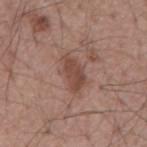Impression:
No biopsy was performed on this lesion — it was imaged during a full skin examination and was not determined to be concerning.
Image and clinical context:
On the mid back. A male subject, aged 53 to 57. Cropped from a whole-body photographic skin survey; the tile spans about 15 mm. An algorithmic analysis of the crop reported an area of roughly 7.5 mm², a shape eccentricity near 0.85, and two-axis asymmetry of about 0.3. The software also gave a mean CIELAB color near L≈46 a*≈20 b*≈25, a lesion–skin lightness drop of about 9, and a normalized lesion–skin contrast near 7.5. It also reported a border-irregularity rating of about 3/10, a color-variation rating of about 2/10, and radial color variation of about 0.5. The lesion's longest dimension is about 4.5 mm.